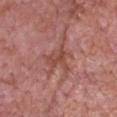Clinical impression: The lesion was photographed on a routine skin check and not biopsied; there is no pathology result. Acquisition and patient details: Measured at roughly 4.5 mm in maximum diameter. A male subject aged around 60. From the head or neck. Imaged with white-light lighting. A roughly 15 mm field-of-view crop from a total-body skin photograph.